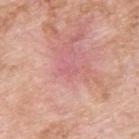The lesion was photographed on a routine skin check and not biopsied; there is no pathology result. About 2 mm across. Imaged with white-light lighting. A male patient, about 60 years old. On the upper back. Automated image analysis of the tile measured a footprint of about 2 mm², an eccentricity of roughly 0.75, and two-axis asymmetry of about 0.35. The software also gave an average lesion color of about L≈61 a*≈30 b*≈21 (CIELAB), about 4 CIELAB-L* units darker than the surrounding skin, and a normalized border contrast of about 3.5. A lesion tile, about 15 mm wide, cut from a 3D total-body photograph.